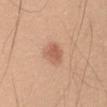Imaged during a routine full-body skin examination; the lesion was not biopsied and no histopathology is available. A roughly 15 mm field-of-view crop from a total-body skin photograph. On the left upper arm. Longest diameter approximately 3 mm. A male subject, approximately 50 years of age. Imaged with white-light lighting.